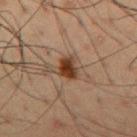Assessment:
Captured during whole-body skin photography for melanoma surveillance; the lesion was not biopsied.
Background:
A male patient, aged around 50. The lesion is located on the chest. This image is a 15 mm lesion crop taken from a total-body photograph.